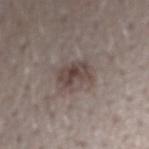Recorded during total-body skin imaging; not selected for excision or biopsy.
Cropped from a whole-body photographic skin survey; the tile spans about 15 mm.
The lesion is on the right forearm.
The lesion's longest dimension is about 3.5 mm.
The patient is a male in their 30s.